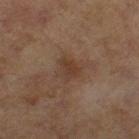The lesion was tiled from a total-body skin photograph and was not biopsied. A female subject, approximately 60 years of age. A roughly 15 mm field-of-view crop from a total-body skin photograph. This is a cross-polarized tile. About 3 mm across. From the left thigh. The total-body-photography lesion software estimated a classifier nevus-likeness of about 5/100 and a detector confidence of about 100 out of 100 that the crop contains a lesion.